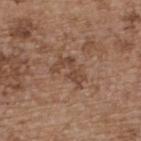workup: imaged on a skin check; not biopsied | illumination: white-light | automated lesion analysis: an area of roughly 6.5 mm², an outline eccentricity of about 0.85 (0 = round, 1 = elongated), and two-axis asymmetry of about 0.55; roughly 8 lightness units darker than nearby skin and a lesion-to-skin contrast of about 6.5 (normalized; higher = more distinct) | diameter: ~4 mm (longest diameter) | body site: the upper back | patient: female, in their mid-60s | imaging modality: 15 mm crop, total-body photography.